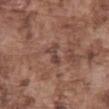{
  "biopsy_status": "not biopsied; imaged during a skin examination",
  "patient": {
    "sex": "male",
    "age_approx": 75
  },
  "image": {
    "source": "total-body photography crop",
    "field_of_view_mm": 15
  },
  "site": "abdomen",
  "automated_metrics": {
    "area_mm2_approx": 3.5,
    "eccentricity": 0.9,
    "cielab_L": 42,
    "cielab_a": 20,
    "cielab_b": 23,
    "vs_skin_darker_L": 8.0,
    "vs_skin_contrast_norm": 7.0,
    "border_irregularity_0_10": 6.0,
    "color_variation_0_10": 0.0,
    "nevus_likeness_0_100": 0,
    "lesion_detection_confidence_0_100": 95
  },
  "lesion_size": {
    "long_diameter_mm_approx": 3.0
  },
  "lighting": "white-light"
}